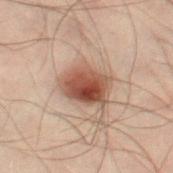follow-up — catalogued during a skin exam; not biopsied
patient — male, roughly 50 years of age
TBP lesion metrics — a lesion area of about 13 mm² and a symmetry-axis asymmetry near 0.2; an average lesion color of about L≈42 a*≈18 b*≈24 (CIELAB), a lesion–skin lightness drop of about 13, and a lesion-to-skin contrast of about 10.5 (normalized; higher = more distinct); a border-irregularity rating of about 2/10 and a peripheral color-asymmetry measure near 2.5; a classifier nevus-likeness of about 100/100 and a detector confidence of about 100 out of 100 that the crop contains a lesion
image source — total-body-photography crop, ~15 mm field of view
anatomic site — the left leg
size — ~4.5 mm (longest diameter)
illumination — cross-polarized